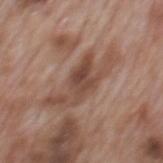Recorded during total-body skin imaging; not selected for excision or biopsy. Imaged with white-light lighting. The lesion is located on the mid back. The recorded lesion diameter is about 8 mm. Automated image analysis of the tile measured a mean CIELAB color near L≈48 a*≈19 b*≈27, a lesion–skin lightness drop of about 10, and a normalized lesion–skin contrast near 7.5. The software also gave a detector confidence of about 55 out of 100 that the crop contains a lesion. A close-up tile cropped from a whole-body skin photograph, about 15 mm across. A male subject, aged around 70.